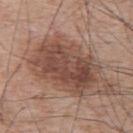workup: catalogued during a skin exam; not biopsied | subject: male, aged around 65 | image: ~15 mm tile from a whole-body skin photo | lighting: white-light | automated metrics: an outline eccentricity of about 0.75 (0 = round, 1 = elongated) and a shape-asymmetry score of about 0.2 (0 = symmetric) | site: the upper back | lesion size: about 8 mm.